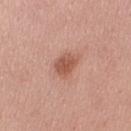Notes:
* notes — total-body-photography surveillance lesion; no biopsy
* location — the right upper arm
* lighting — white-light illumination
* automated lesion analysis — an area of roughly 6 mm², an outline eccentricity of about 0.7 (0 = round, 1 = elongated), and two-axis asymmetry of about 0.25; a lesion color around L≈55 a*≈25 b*≈30 in CIELAB and roughly 11 lightness units darker than nearby skin; a classifier nevus-likeness of about 80/100 and a lesion-detection confidence of about 100/100
* subject — female, in their mid- to late 20s
* imaging modality — ~15 mm crop, total-body skin-cancer survey